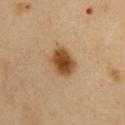  biopsy_status: not biopsied; imaged during a skin examination
  lighting: cross-polarized
  site: abdomen
  patient:
    sex: male
    age_approx: 50
  automated_metrics:
    eccentricity: 0.7
    shape_asymmetry: 0.15
    cielab_L: 48
    cielab_a: 21
    cielab_b: 37
    vs_skin_darker_L: 15.0
  lesion_size:
    long_diameter_mm_approx: 4.0
  image:
    source: total-body photography crop
    field_of_view_mm: 15